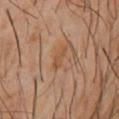Captured during whole-body skin photography for melanoma surveillance; the lesion was not biopsied. A 15 mm crop from a total-body photograph taken for skin-cancer surveillance. On the chest. The subject is a male in their 60s.